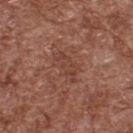follow-up=imaged on a skin check; not biopsied
patient=male, approximately 75 years of age
acquisition=15 mm crop, total-body photography
body site=the back